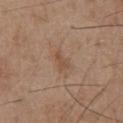<record>
  <biopsy_status>not biopsied; imaged during a skin examination</biopsy_status>
  <site>abdomen</site>
  <patient>
    <sex>male</sex>
    <age_approx>55</age_approx>
  </patient>
  <image>
    <source>total-body photography crop</source>
    <field_of_view_mm>15</field_of_view_mm>
  </image>
  <automated_metrics>
    <area_mm2_approx>2.5</area_mm2_approx>
    <eccentricity>0.9</eccentricity>
    <shape_asymmetry>0.4</shape_asymmetry>
    <vs_skin_darker_L>7.0</vs_skin_darker_L>
    <vs_skin_contrast_norm>6.0</vs_skin_contrast_norm>
  </automated_metrics>
</record>